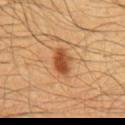Clinical impression:
Imaged during a routine full-body skin examination; the lesion was not biopsied and no histopathology is available.
Background:
A roughly 15 mm field-of-view crop from a total-body skin photograph. Located on the chest. Measured at roughly 3.5 mm in maximum diameter. The total-body-photography lesion software estimated a lesion area of about 6 mm², an eccentricity of roughly 0.75, and two-axis asymmetry of about 0.25. The analysis additionally found a classifier nevus-likeness of about 100/100. A male subject, aged 58–62. Imaged with cross-polarized lighting.